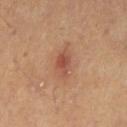Part of a total-body skin-imaging series; this lesion was reviewed on a skin check and was not flagged for biopsy.
A female patient in their 40s.
The recorded lesion diameter is about 3.5 mm.
This is a cross-polarized tile.
From the left thigh.
A 15 mm close-up tile from a total-body photography series done for melanoma screening.